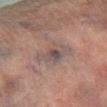workup = imaged on a skin check; not biopsied
image source = 15 mm crop, total-body photography
patient = male, aged approximately 75
body site = the right lower leg
tile lighting = cross-polarized
size = about 4.5 mm